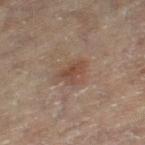| field | value |
|---|---|
| workup | catalogued during a skin exam; not biopsied |
| subject | female, aged approximately 80 |
| imaging modality | ~15 mm crop, total-body skin-cancer survey |
| lesion diameter | ~3 mm (longest diameter) |
| tile lighting | cross-polarized |
| anatomic site | the right leg |
| automated metrics | a footprint of about 4.5 mm² and an eccentricity of roughly 0.85; border irregularity of about 4 on a 0–10 scale, a within-lesion color-variation index near 2.5/10, and peripheral color asymmetry of about 0.5; a nevus-likeness score of about 10/100 and a lesion-detection confidence of about 100/100 |